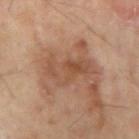Case summary:
- follow-up · imaged on a skin check; not biopsied
- diameter · ≈5.5 mm
- location · the right upper arm
- imaging modality · 15 mm crop, total-body photography
- patient · male, aged around 65